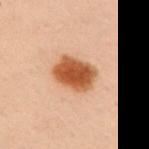notes — catalogued during a skin exam; not biopsied | body site — the chest | lesion diameter — ~4.5 mm (longest diameter) | subject — female, in their 50s | acquisition — ~15 mm tile from a whole-body skin photo | lighting — cross-polarized illumination.